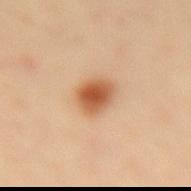biopsy_status: not biopsied; imaged during a skin examination
lesion_size:
  long_diameter_mm_approx: 3.5
image:
  source: total-body photography crop
  field_of_view_mm: 15
site: lower back
patient:
  sex: female
  age_approx: 50
lighting: cross-polarized